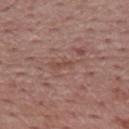| field | value |
|---|---|
| biopsy status | catalogued during a skin exam; not biopsied |
| lighting | white-light illumination |
| patient | male, roughly 55 years of age |
| anatomic site | the mid back |
| acquisition | 15 mm crop, total-body photography |